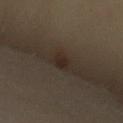{"automated_metrics": {"eccentricity": 0.8, "shape_asymmetry": 0.35, "cielab_L": 25, "cielab_a": 12, "cielab_b": 19, "vs_skin_darker_L": 7.0, "vs_skin_contrast_norm": 8.5}, "image": {"source": "total-body photography crop", "field_of_view_mm": 15}, "lesion_size": {"long_diameter_mm_approx": 2.5}, "site": "right upper arm", "patient": {"sex": "female", "age_approx": 55}, "lighting": "cross-polarized"}